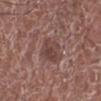<tbp_lesion>
<lighting>white-light</lighting>
<image>
  <source>total-body photography crop</source>
  <field_of_view_mm>15</field_of_view_mm>
</image>
<automated_metrics>
  <border_irregularity_0_10>1.5</border_irregularity_0_10>
  <color_variation_0_10>3.0</color_variation_0_10>
  <peripheral_color_asymmetry>1.0</peripheral_color_asymmetry>
</automated_metrics>
<patient>
  <sex>male</sex>
  <age_approx>75</age_approx>
</patient>
<site>left lower leg</site>
</tbp_lesion>